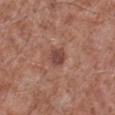workup: catalogued during a skin exam; not biopsied | subject: male, aged 53 to 57 | anatomic site: the left lower leg | size: ≈2.5 mm | imaging modality: total-body-photography crop, ~15 mm field of view.